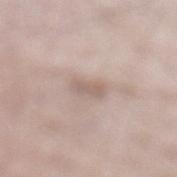Clinical impression: The lesion was photographed on a routine skin check and not biopsied; there is no pathology result. Context: The lesion-visualizer software estimated a lesion color around L≈61 a*≈15 b*≈23 in CIELAB, about 8 CIELAB-L* units darker than the surrounding skin, and a normalized lesion–skin contrast near 5.5. On the leg. A male patient, aged around 50. Cropped from a whole-body photographic skin survey; the tile spans about 15 mm. Measured at roughly 2.5 mm in maximum diameter. Imaged with white-light lighting.